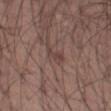workup = no biopsy performed (imaged during a skin exam) | lighting = white-light | TBP lesion metrics = a lesion color around L≈41 a*≈17 b*≈21 in CIELAB and roughly 7 lightness units darker than nearby skin; border irregularity of about 4.5 on a 0–10 scale, internal color variation of about 0 on a 0–10 scale, and radial color variation of about 0; a detector confidence of about 95 out of 100 that the crop contains a lesion | patient = male, aged around 55 | site = the left thigh | image source = ~15 mm crop, total-body skin-cancer survey | lesion diameter = about 2.5 mm.